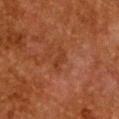Clinical impression:
Captured during whole-body skin photography for melanoma surveillance; the lesion was not biopsied.
Context:
A female patient, approximately 50 years of age. A region of skin cropped from a whole-body photographic capture, roughly 15 mm wide. Measured at roughly 2.5 mm in maximum diameter. From the front of the torso.